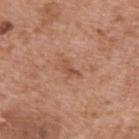Clinical impression: The lesion was photographed on a routine skin check and not biopsied; there is no pathology result. Clinical summary: Cropped from a whole-body photographic skin survey; the tile spans about 15 mm. The lesion is on the upper back. Captured under white-light illumination. A male patient, roughly 60 years of age. Approximately 2.5 mm at its widest.